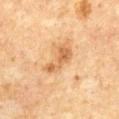notes: imaged on a skin check; not biopsied | image-analysis metrics: an area of roughly 6.5 mm², an outline eccentricity of about 0.9 (0 = round, 1 = elongated), and a symmetry-axis asymmetry near 0.45; an average lesion color of about L≈57 a*≈21 b*≈39 (CIELAB), about 10 CIELAB-L* units darker than the surrounding skin, and a normalized lesion–skin contrast near 7; a border-irregularity index near 5.5/10, internal color variation of about 2 on a 0–10 scale, and radial color variation of about 0.5 | location: the chest | lesion diameter: ~4.5 mm (longest diameter) | subject: male, aged 63–67 | imaging modality: ~15 mm tile from a whole-body skin photo | tile lighting: cross-polarized.